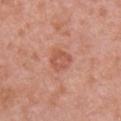The lesion was tiled from a total-body skin photograph and was not biopsied.